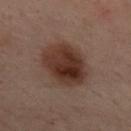Imaged during a routine full-body skin examination; the lesion was not biopsied and no histopathology is available. Captured under cross-polarized illumination. The total-body-photography lesion software estimated a lesion area of about 22 mm² and an outline eccentricity of about 0.8 (0 = round, 1 = elongated). It also reported a color-variation rating of about 5.5/10. It also reported an automated nevus-likeness rating near 95 out of 100. This image is a 15 mm lesion crop taken from a total-body photograph. Located on the upper back. The patient is a female about 55 years old.